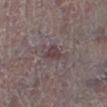No biopsy was performed on this lesion — it was imaged during a full skin examination and was not determined to be concerning.
Cropped from a whole-body photographic skin survey; the tile spans about 15 mm.
Imaged with white-light lighting.
The subject is a male roughly 80 years of age.
From the left lower leg.
An algorithmic analysis of the crop reported a mean CIELAB color near L≈40 a*≈16 b*≈12, a lesion–skin lightness drop of about 8, and a lesion-to-skin contrast of about 7.5 (normalized; higher = more distinct). The software also gave a within-lesion color-variation index near 2/10.